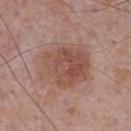workup=catalogued during a skin exam; not biopsied | subject=male, in their mid- to late 70s | lesion diameter=≈6.5 mm | site=the chest | lighting=white-light illumination | imaging modality=~15 mm crop, total-body skin-cancer survey.